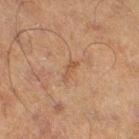Assessment:
Captured during whole-body skin photography for melanoma surveillance; the lesion was not biopsied.
Background:
A male patient roughly 70 years of age. A 15 mm close-up extracted from a 3D total-body photography capture. From the right thigh. Approximately 2.5 mm at its widest.